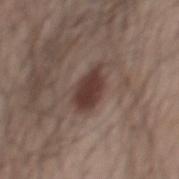- size — ~4.5 mm (longest diameter)
- image source — ~15 mm crop, total-body skin-cancer survey
- subject — male, aged 58–62
- site — the back
- illumination — white-light illumination